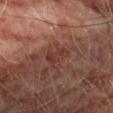biopsy status = catalogued during a skin exam; not biopsied
image-analysis metrics = a footprint of about 4.5 mm² and a shape eccentricity near 0.75; a lesion color around L≈28 a*≈19 b*≈20 in CIELAB, a lesion–skin lightness drop of about 5, and a normalized border contrast of about 5.5; an automated nevus-likeness rating near 0 out of 100 and lesion-presence confidence of about 90/100
lighting = cross-polarized
patient = male, aged 73–77
lesion size = ≈3 mm
imaging modality = total-body-photography crop, ~15 mm field of view
location = the left thigh